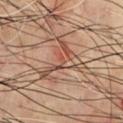No biopsy was performed on this lesion — it was imaged during a full skin examination and was not determined to be concerning.
A region of skin cropped from a whole-body photographic capture, roughly 15 mm wide.
Longest diameter approximately 6 mm.
A male patient aged 68 to 72.
Located on the chest.
This is a cross-polarized tile.